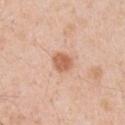patient — male, in their 50s
automated lesion analysis — a border-irregularity index near 2/10, a within-lesion color-variation index near 2/10, and radial color variation of about 0.5
imaging modality — ~15 mm tile from a whole-body skin photo
anatomic site — the right upper arm
lighting — white-light